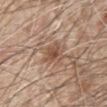Image and clinical context:
The lesion is located on the front of the torso. The patient is a male aged 78 to 82. Automated tile analysis of the lesion measured a footprint of about 5.5 mm², an eccentricity of roughly 0.5, and a shape-asymmetry score of about 0.35 (0 = symmetric). It also reported a classifier nevus-likeness of about 75/100 and a lesion-detection confidence of about 100/100. A 15 mm close-up extracted from a 3D total-body photography capture.